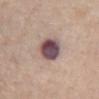Part of a total-body skin-imaging series; this lesion was reviewed on a skin check and was not flagged for biopsy.
A 15 mm crop from a total-body photograph taken for skin-cancer surveillance.
A female subject aged approximately 65.
Located on the abdomen.